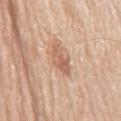| feature | finding |
|---|---|
| subject | male, aged around 80 |
| anatomic site | the mid back |
| imaging modality | ~15 mm crop, total-body skin-cancer survey |